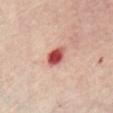Case summary:
• notes — catalogued during a skin exam; not biopsied
• automated metrics — an area of roughly 5.5 mm² and an outline eccentricity of about 0.65 (0 = round, 1 = elongated); about 19 CIELAB-L* units darker than the surrounding skin and a normalized lesion–skin contrast near 12; a border-irregularity index near 2/10 and radial color variation of about 1.5; an automated nevus-likeness rating near 0 out of 100 and a detector confidence of about 100 out of 100 that the crop contains a lesion
• location — the chest
• lesion size — ≈3 mm
• image — ~15 mm crop, total-body skin-cancer survey
• illumination — cross-polarized
• patient — female, approximately 60 years of age